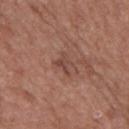Assessment:
Captured during whole-body skin photography for melanoma surveillance; the lesion was not biopsied.
Acquisition and patient details:
A lesion tile, about 15 mm wide, cut from a 3D total-body photograph. On the mid back. Automated image analysis of the tile measured a lesion–skin lightness drop of about 8 and a normalized border contrast of about 6. The software also gave a border-irregularity rating of about 4.5/10, internal color variation of about 3.5 on a 0–10 scale, and radial color variation of about 1.5. The software also gave an automated nevus-likeness rating near 0 out of 100. About 3 mm across. The patient is a male aged 48–52.